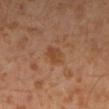* follow-up: total-body-photography surveillance lesion; no biopsy
* image: 15 mm crop, total-body photography
* anatomic site: the left lower leg
* lesion size: about 2.5 mm
* patient: male, aged 28 to 32